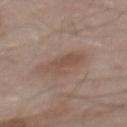No biopsy was performed on this lesion — it was imaged during a full skin examination and was not determined to be concerning. The lesion is on the mid back. This is a white-light tile. The subject is a male about 55 years old. An algorithmic analysis of the crop reported a footprint of about 8.5 mm², an eccentricity of roughly 0.9, and two-axis asymmetry of about 0.25. It also reported a mean CIELAB color near L≈50 a*≈16 b*≈25, roughly 8 lightness units darker than nearby skin, and a lesion-to-skin contrast of about 6 (normalized; higher = more distinct). The software also gave border irregularity of about 4 on a 0–10 scale, a color-variation rating of about 2.5/10, and peripheral color asymmetry of about 1. The lesion's longest dimension is about 4.5 mm. This image is a 15 mm lesion crop taken from a total-body photograph.